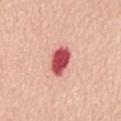| feature | finding |
|---|---|
| follow-up | total-body-photography surveillance lesion; no biopsy |
| image | total-body-photography crop, ~15 mm field of view |
| patient | female, aged around 70 |
| location | the back |